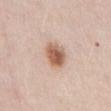{"biopsy_status": "not biopsied; imaged during a skin examination", "image": {"source": "total-body photography crop", "field_of_view_mm": 15}, "patient": {"sex": "male", "age_approx": 40}, "lighting": "white-light", "site": "abdomen", "lesion_size": {"long_diameter_mm_approx": 3.5}, "automated_metrics": {"cielab_L": 60, "cielab_a": 21, "cielab_b": 29}}